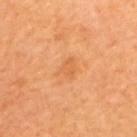{"biopsy_status": "not biopsied; imaged during a skin examination", "automated_metrics": {"cielab_L": 62, "cielab_a": 27, "cielab_b": 45, "vs_skin_darker_L": 6.0, "vs_skin_contrast_norm": 4.5, "border_irregularity_0_10": 3.5, "color_variation_0_10": 1.5, "peripheral_color_asymmetry": 0.5}, "site": "upper back", "image": {"source": "total-body photography crop", "field_of_view_mm": 15}, "patient": {"sex": "female", "age_approx": 45}, "lighting": "cross-polarized"}